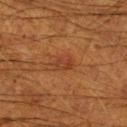Impression: Recorded during total-body skin imaging; not selected for excision or biopsy. Background: The subject is a male in their 60s. The lesion is on the right lower leg. Approximately 2.5 mm at its widest. This image is a 15 mm lesion crop taken from a total-body photograph.